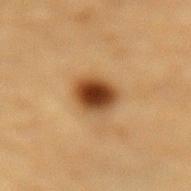{"biopsy_status": "not biopsied; imaged during a skin examination", "automated_metrics": {"eccentricity": 0.55, "shape_asymmetry": 0.15, "color_variation_0_10": 7.0, "peripheral_color_asymmetry": 1.5, "nevus_likeness_0_100": 100, "lesion_detection_confidence_0_100": 100}, "lesion_size": {"long_diameter_mm_approx": 4.0}, "site": "lower back", "patient": {"sex": "male", "age_approx": "85+"}, "image": {"source": "total-body photography crop", "field_of_view_mm": 15}, "lighting": "cross-polarized"}